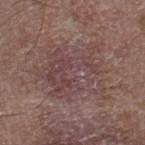workup=total-body-photography surveillance lesion; no biopsy | size=≈7.5 mm | image-analysis metrics=a mean CIELAB color near L≈44 a*≈18 b*≈18, a lesion–skin lightness drop of about 6, and a normalized border contrast of about 5; an automated nevus-likeness rating near 0 out of 100 and a detector confidence of about 85 out of 100 that the crop contains a lesion | anatomic site=the right lower leg | subject=male, aged 48–52 | lighting=white-light | image=total-body-photography crop, ~15 mm field of view.